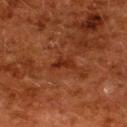{"site": "upper back", "image": {"source": "total-body photography crop", "field_of_view_mm": 15}, "lesion_size": {"long_diameter_mm_approx": 2.5}, "patient": {"sex": "female", "age_approx": 50}}